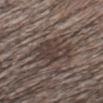Impression: The lesion was photographed on a routine skin check and not biopsied; there is no pathology result. Image and clinical context: A roughly 15 mm field-of-view crop from a total-body skin photograph. Located on the head or neck. A male patient, aged approximately 40. Automated tile analysis of the lesion measured an average lesion color of about L≈37 a*≈11 b*≈17 (CIELAB) and about 9 CIELAB-L* units darker than the surrounding skin. The tile uses white-light illumination. About 6 mm across.